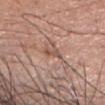workup = total-body-photography surveillance lesion; no biopsy
imaging modality = ~15 mm tile from a whole-body skin photo
location = the head or neck
size = about 2.5 mm
patient = female, in their mid-60s